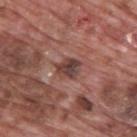About 3 mm across. Cropped from a whole-body photographic skin survey; the tile spans about 15 mm. A male patient aged approximately 70. The lesion-visualizer software estimated a border-irregularity rating of about 2.5/10 and peripheral color asymmetry of about 2. The analysis additionally found a classifier nevus-likeness of about 0/100 and a lesion-detection confidence of about 100/100. The lesion is located on the upper back.